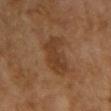Imaged during a routine full-body skin examination; the lesion was not biopsied and no histopathology is available. The lesion is located on the front of the torso. The tile uses cross-polarized illumination. Approximately 5 mm at its widest. The subject is a female about 60 years old. Cropped from a whole-body photographic skin survey; the tile spans about 15 mm. An algorithmic analysis of the crop reported a footprint of about 11 mm² and an eccentricity of roughly 0.85. The analysis additionally found a lesion color around L≈33 a*≈17 b*≈28 in CIELAB, about 7 CIELAB-L* units darker than the surrounding skin, and a lesion-to-skin contrast of about 6.5 (normalized; higher = more distinct). It also reported a detector confidence of about 100 out of 100 that the crop contains a lesion.